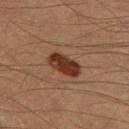The lesion was photographed on a routine skin check and not biopsied; there is no pathology result. About 4 mm across. Automated tile analysis of the lesion measured a classifier nevus-likeness of about 95/100 and a detector confidence of about 100 out of 100 that the crop contains a lesion. The patient is a male roughly 40 years of age. From the left thigh. This is a cross-polarized tile. A 15 mm close-up extracted from a 3D total-body photography capture.